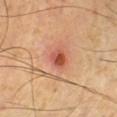This lesion was catalogued during total-body skin photography and was not selected for biopsy. Cropped from a total-body skin-imaging series; the visible field is about 15 mm. The total-body-photography lesion software estimated a border-irregularity rating of about 1.5/10 and a within-lesion color-variation index near 7.5/10. The subject is a male roughly 55 years of age. The tile uses cross-polarized illumination. Longest diameter approximately 2.5 mm. The lesion is located on the leg.